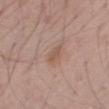Q: Was a biopsy performed?
A: catalogued during a skin exam; not biopsied